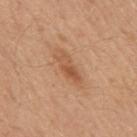Imaged during a routine full-body skin examination; the lesion was not biopsied and no histopathology is available. A male patient aged around 50. Automated tile analysis of the lesion measured a footprint of about 13 mm². And it measured a mean CIELAB color near L≈58 a*≈21 b*≈34, about 7 CIELAB-L* units darker than the surrounding skin, and a lesion-to-skin contrast of about 5 (normalized; higher = more distinct). The analysis additionally found a border-irregularity rating of about 4/10, a within-lesion color-variation index near 5/10, and radial color variation of about 1.5. About 6.5 mm across. A 15 mm close-up extracted from a 3D total-body photography capture. The lesion is located on the mid back. Captured under white-light illumination.